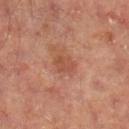Findings:
- subject · male, approximately 65 years of age
- size · ≈2.5 mm
- automated metrics · an area of roughly 4 mm², a shape eccentricity near 0.7, and two-axis asymmetry of about 0.4; a lesion color around L≈49 a*≈25 b*≈31 in CIELAB; border irregularity of about 3.5 on a 0–10 scale and peripheral color asymmetry of about 0.5
- imaging modality · 15 mm crop, total-body photography
- anatomic site · the right lower leg